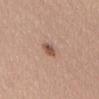{"biopsy_status": "not biopsied; imaged during a skin examination", "patient": {"sex": "female", "age_approx": 45}, "lighting": "white-light", "site": "back", "automated_metrics": {"area_mm2_approx": 3.0, "shape_asymmetry": 0.25, "border_irregularity_0_10": 2.5, "color_variation_0_10": 3.5}, "image": {"source": "total-body photography crop", "field_of_view_mm": 15}}